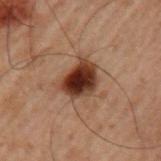Imaged during a routine full-body skin examination; the lesion was not biopsied and no histopathology is available. The lesion is on the left upper arm. Cropped from a total-body skin-imaging series; the visible field is about 15 mm. A male patient aged 58 to 62. Automated tile analysis of the lesion measured a mean CIELAB color near L≈25 a*≈18 b*≈22, a lesion–skin lightness drop of about 16, and a normalized lesion–skin contrast near 15.5. The analysis additionally found a nevus-likeness score of about 100/100. The recorded lesion diameter is about 4 mm. Imaged with cross-polarized lighting.